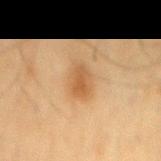Case summary:
• workup: total-body-photography surveillance lesion; no biopsy